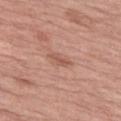No biopsy was performed on this lesion — it was imaged during a full skin examination and was not determined to be concerning.
The subject is a female approximately 70 years of age.
A 15 mm close-up tile from a total-body photography series done for melanoma screening.
The lesion is located on the left thigh.